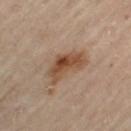The lesion was tiled from a total-body skin photograph and was not biopsied. Located on the left thigh. The tile uses cross-polarized illumination. Cropped from a whole-body photographic skin survey; the tile spans about 15 mm. A female patient in their 60s. Approximately 5 mm at its widest.